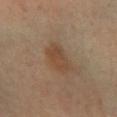  biopsy_status: not biopsied; imaged during a skin examination
  image:
    source: total-body photography crop
    field_of_view_mm: 15
  patient:
    sex: female
    age_approx: 30
  site: right lower leg
  lighting: cross-polarized
  lesion_size:
    long_diameter_mm_approx: 4.0